Cropped from a whole-body photographic skin survey; the tile spans about 15 mm; a female patient aged approximately 60; on the left leg: 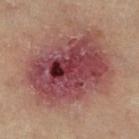Background:
The recorded lesion diameter is about 10 mm. Automated image analysis of the tile measured a footprint of about 60 mm², an eccentricity of roughly 0.65, and a symmetry-axis asymmetry near 0.15. It also reported border irregularity of about 2.5 on a 0–10 scale. It also reported a classifier nevus-likeness of about 0/100 and a lesion-detection confidence of about 100/100. This is a cross-polarized tile.
Diagnosis:
Histopathological examination showed a superficial basal cell carcinoma — a malignant lesion.